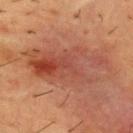This lesion was catalogued during total-body skin photography and was not selected for biopsy. On the front of the torso. Approximately 10 mm at its widest. A male subject roughly 55 years of age. A close-up tile cropped from a whole-body skin photograph, about 15 mm across.